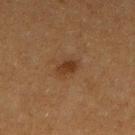Context:
The recorded lesion diameter is about 2.5 mm. A region of skin cropped from a whole-body photographic capture, roughly 15 mm wide. The patient is a female aged around 40. Automated tile analysis of the lesion measured an eccentricity of roughly 0.75 and two-axis asymmetry of about 0.2. The analysis additionally found a border-irregularity rating of about 2/10 and a color-variation rating of about 2/10. And it measured an automated nevus-likeness rating near 65 out of 100 and a detector confidence of about 100 out of 100 that the crop contains a lesion. The lesion is on the arm.